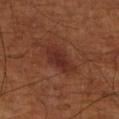Image and clinical context:
The lesion is located on the leg. Approximately 4 mm at its widest. A 15 mm close-up tile from a total-body photography series done for melanoma screening. A male patient, aged 68–72. Imaged with cross-polarized lighting.